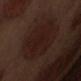Q: Is there a histopathology result?
A: imaged on a skin check; not biopsied
Q: Lesion location?
A: the abdomen
Q: Automated lesion metrics?
A: a lesion area of about 30 mm², an eccentricity of roughly 0.5, and two-axis asymmetry of about 0.1; a lesion color around L≈18 a*≈17 b*≈18 in CIELAB and roughly 5 lightness units darker than nearby skin; a peripheral color-asymmetry measure near 1; an automated nevus-likeness rating near 45 out of 100 and a detector confidence of about 100 out of 100 that the crop contains a lesion
Q: How was this image acquired?
A: ~15 mm crop, total-body skin-cancer survey
Q: How large is the lesion?
A: ≈7 mm
Q: What are the patient's age and sex?
A: male, aged 68 to 72